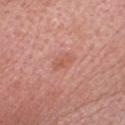workup: no biopsy performed (imaged during a skin exam) | acquisition: ~15 mm tile from a whole-body skin photo | body site: the head or neck | subject: female, aged around 65.